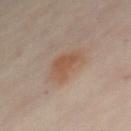Imaged during a routine full-body skin examination; the lesion was not biopsied and no histopathology is available.
Cropped from a total-body skin-imaging series; the visible field is about 15 mm.
This is a cross-polarized tile.
A female patient, about 60 years old.
Measured at roughly 4 mm in maximum diameter.
The lesion is on the front of the torso.